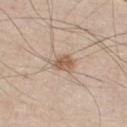notes — total-body-photography surveillance lesion; no biopsy
patient — male, approximately 45 years of age
diameter — ≈3 mm
acquisition — ~15 mm tile from a whole-body skin photo
location — the chest